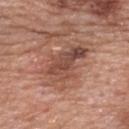Q: Is there a histopathology result?
A: imaged on a skin check; not biopsied